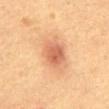  image:
    source: total-body photography crop
    field_of_view_mm: 15
  patient:
    sex: male
    age_approx: 50
  site: front of the torso
  lesion_size:
    long_diameter_mm_approx: 4.0
  lighting: cross-polarized